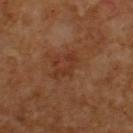Clinical summary:
A region of skin cropped from a whole-body photographic capture, roughly 15 mm wide. On the back. The subject is a male roughly 60 years of age.